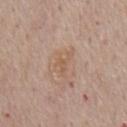Findings:
• biopsy status · no biopsy performed (imaged during a skin exam)
• automated metrics · a lesion color around L≈58 a*≈17 b*≈30 in CIELAB, a lesion–skin lightness drop of about 6, and a normalized border contrast of about 5; a nevus-likeness score of about 0/100 and a lesion-detection confidence of about 100/100
• illumination · white-light illumination
• patient · male, roughly 75 years of age
• lesion diameter · about 3 mm
• site · the chest
• image · ~15 mm crop, total-body skin-cancer survey